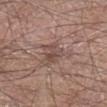Acquisition and patient details:
A male patient aged 58–62. The lesion-visualizer software estimated a lesion color around L≈48 a*≈17 b*≈22 in CIELAB and about 8 CIELAB-L* units darker than the surrounding skin. The software also gave a border-irregularity index near 3.5/10, internal color variation of about 4.5 on a 0–10 scale, and radial color variation of about 1.5. From the right lower leg. Captured under white-light illumination. The recorded lesion diameter is about 3 mm. A 15 mm close-up tile from a total-body photography series done for melanoma screening.